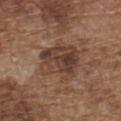The lesion was tiled from a total-body skin photograph and was not biopsied.
A roughly 15 mm field-of-view crop from a total-body skin photograph.
The tile uses white-light illumination.
The patient is a male aged around 75.
The lesion is located on the chest.
Measured at roughly 5 mm in maximum diameter.